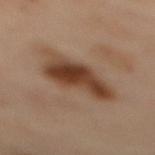{
  "biopsy_status": "not biopsied; imaged during a skin examination",
  "image": {
    "source": "total-body photography crop",
    "field_of_view_mm": 15
  },
  "patient": {
    "sex": "female",
    "age_approx": 55
  },
  "site": "back"
}